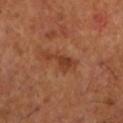lighting: cross-polarized
patient:
  sex: male
  age_approx: 65
image:
  source: total-body photography crop
  field_of_view_mm: 15
lesion_size:
  long_diameter_mm_approx: 5.0
site: left lower leg
automated_metrics:
  area_mm2_approx: 6.0
  eccentricity: 0.95
  vs_skin_darker_L: 8.0
  vs_skin_contrast_norm: 6.5